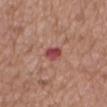A 15 mm crop from a total-body photograph taken for skin-cancer surveillance. A female subject, aged 73–77. The lesion is located on the back. Longest diameter approximately 2.5 mm. Automated tile analysis of the lesion measured a footprint of about 4 mm², a shape eccentricity near 0.7, and a symmetry-axis asymmetry near 0.15. It also reported a classifier nevus-likeness of about 0/100 and lesion-presence confidence of about 100/100. Imaged with white-light lighting.